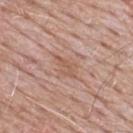Notes:
– notes — imaged on a skin check; not biopsied
– lesion diameter — ≈3 mm
– illumination — white-light
– subject — male, in their mid-60s
– image — 15 mm crop, total-body photography
– location — the mid back